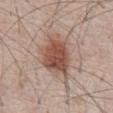Case summary:
• follow-up — total-body-photography surveillance lesion; no biopsy
• anatomic site — the chest
• subject — male, approximately 65 years of age
• automated lesion analysis — a shape eccentricity near 0.75 and a symmetry-axis asymmetry near 0.2; a border-irregularity rating of about 3/10 and internal color variation of about 5.5 on a 0–10 scale
• image source — ~15 mm crop, total-body skin-cancer survey
• illumination — white-light